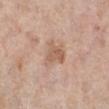Assessment: Part of a total-body skin-imaging series; this lesion was reviewed on a skin check and was not flagged for biopsy. Clinical summary: Located on the right lower leg. Longest diameter approximately 3 mm. Automated tile analysis of the lesion measured a border-irregularity index near 4.5/10 and peripheral color asymmetry of about 1. Cropped from a total-body skin-imaging series; the visible field is about 15 mm. A female patient, roughly 65 years of age.